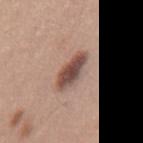Q: Was a biopsy performed?
A: imaged on a skin check; not biopsied